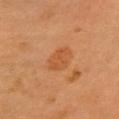{"biopsy_status": "not biopsied; imaged during a skin examination", "automated_metrics": {"eccentricity": 0.45, "shape_asymmetry": 0.2, "nevus_likeness_0_100": 40, "lesion_detection_confidence_0_100": 100}, "patient": {"sex": "male", "age_approx": 60}, "image": {"source": "total-body photography crop", "field_of_view_mm": 15}, "site": "head or neck"}